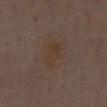  biopsy_status: not biopsied; imaged during a skin examination
  lesion_size:
    long_diameter_mm_approx: 4.5
  lighting: white-light
  image:
    source: total-body photography crop
    field_of_view_mm: 15
  patient:
    sex: male
    age_approx: 70
  site: chest